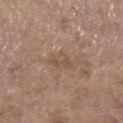notes = no biopsy performed (imaged during a skin exam)
image source = 15 mm crop, total-body photography
illumination = white-light illumination
patient = female, aged approximately 65
automated lesion analysis = a lesion area of about 4 mm²; an average lesion color of about L≈51 a*≈17 b*≈28 (CIELAB), about 6 CIELAB-L* units darker than the surrounding skin, and a normalized border contrast of about 5; a color-variation rating of about 2/10 and peripheral color asymmetry of about 0.5; a nevus-likeness score of about 0/100 and a lesion-detection confidence of about 100/100
location = the right lower leg
diameter = ≈3 mm